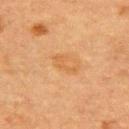Recorded during total-body skin imaging; not selected for excision or biopsy. The total-body-photography lesion software estimated an area of roughly 3 mm² and a shape eccentricity near 0.9. The analysis additionally found an average lesion color of about L≈49 a*≈19 b*≈36 (CIELAB), a lesion–skin lightness drop of about 5, and a normalized border contrast of about 5. The software also gave a border-irregularity rating of about 6.5/10, a color-variation rating of about 0/10, and a peripheral color-asymmetry measure near 0. From the upper back. A lesion tile, about 15 mm wide, cut from a 3D total-body photograph. A female patient, aged 53 to 57. Approximately 3 mm at its widest. The tile uses cross-polarized illumination.